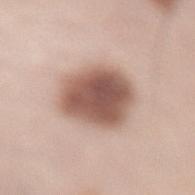notes: catalogued during a skin exam; not biopsied
size: about 5.5 mm
lighting: white-light
location: the lower back
image: ~15 mm tile from a whole-body skin photo
subject: female, about 50 years old